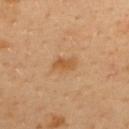Recorded during total-body skin imaging; not selected for excision or biopsy. A 15 mm crop from a total-body photograph taken for skin-cancer surveillance. Automated image analysis of the tile measured a lesion area of about 4 mm², an outline eccentricity of about 0.8 (0 = round, 1 = elongated), and a shape-asymmetry score of about 0.3 (0 = symmetric). It also reported an average lesion color of about L≈56 a*≈21 b*≈40 (CIELAB), about 8 CIELAB-L* units darker than the surrounding skin, and a lesion-to-skin contrast of about 6.5 (normalized; higher = more distinct). A male patient, aged 38 to 42. Approximately 2.5 mm at its widest. Located on the back.